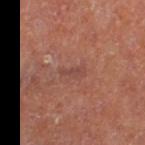| field | value |
|---|---|
| notes | imaged on a skin check; not biopsied |
| TBP lesion metrics | a footprint of about 2.5 mm², an outline eccentricity of about 0.9 (0 = round, 1 = elongated), and a shape-asymmetry score of about 0.4 (0 = symmetric); a nevus-likeness score of about 0/100 and a detector confidence of about 90 out of 100 that the crop contains a lesion |
| diameter | ≈2.5 mm |
| acquisition | total-body-photography crop, ~15 mm field of view |
| illumination | cross-polarized |
| location | the left thigh |
| subject | female |